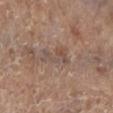Assessment:
Imaged during a routine full-body skin examination; the lesion was not biopsied and no histopathology is available.
Acquisition and patient details:
This image is a 15 mm lesion crop taken from a total-body photograph. A female subject about 85 years old or older. The lesion is located on the left lower leg.